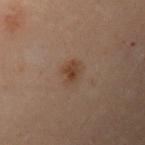Image and clinical context:
From the left upper arm. The lesion-visualizer software estimated a mean CIELAB color near L≈33 a*≈14 b*≈24, a lesion–skin lightness drop of about 6, and a lesion-to-skin contrast of about 7 (normalized; higher = more distinct). It also reported a nevus-likeness score of about 80/100 and a detector confidence of about 100 out of 100 that the crop contains a lesion. Imaged with cross-polarized lighting. A female patient roughly 30 years of age. A roughly 15 mm field-of-view crop from a total-body skin photograph. About 2.5 mm across.